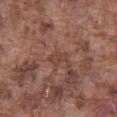Case summary:
- follow-up — no biopsy performed (imaged during a skin exam)
- size — about 2.5 mm
- image source — ~15 mm tile from a whole-body skin photo
- illumination — white-light
- patient — male, about 75 years old
- anatomic site — the abdomen